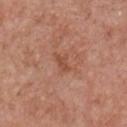Q: Is there a histopathology result?
A: imaged on a skin check; not biopsied
Q: What kind of image is this?
A: 15 mm crop, total-body photography
Q: What is the anatomic site?
A: the chest
Q: Who is the patient?
A: male, in their 60s